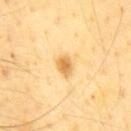Clinical impression:
No biopsy was performed on this lesion — it was imaged during a full skin examination and was not determined to be concerning.
Image and clinical context:
From the chest. Cropped from a total-body skin-imaging series; the visible field is about 15 mm. A male patient aged 63 to 67.